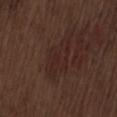| key | value |
|---|---|
| follow-up | total-body-photography surveillance lesion; no biopsy |
| TBP lesion metrics | a mean CIELAB color near L≈24 a*≈17 b*≈20, roughly 5 lightness units darker than nearby skin, and a normalized border contrast of about 5.5 |
| body site | the lower back |
| size | ≈5.5 mm |
| illumination | white-light illumination |
| imaging modality | 15 mm crop, total-body photography |
| patient | male, aged 68–72 |